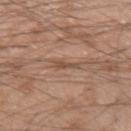notes: no biopsy performed (imaged during a skin exam) | TBP lesion metrics: an average lesion color of about L≈50 a*≈18 b*≈28 (CIELAB), roughly 8 lightness units darker than nearby skin, and a lesion-to-skin contrast of about 6 (normalized; higher = more distinct); border irregularity of about 4 on a 0–10 scale, a within-lesion color-variation index near 0/10, and radial color variation of about 0; a detector confidence of about 60 out of 100 that the crop contains a lesion | imaging modality: 15 mm crop, total-body photography | patient: male, roughly 20 years of age | lighting: white-light | site: the left upper arm | lesion diameter: about 2.5 mm.